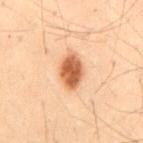biopsy status — imaged on a skin check; not biopsied | lesion size — ≈4 mm | location — the lower back | illumination — cross-polarized illumination | subject — male, aged 28–32 | acquisition — total-body-photography crop, ~15 mm field of view.